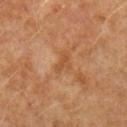Notes:
- subject — male, aged around 55
- body site — the left forearm
- diameter — ≈2.5 mm
- image-analysis metrics — a mean CIELAB color near L≈50 a*≈24 b*≈38, roughly 7 lightness units darker than nearby skin, and a lesion-to-skin contrast of about 5.5 (normalized; higher = more distinct); a peripheral color-asymmetry measure near 0
- imaging modality — 15 mm crop, total-body photography